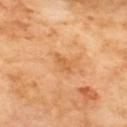follow-up: total-body-photography surveillance lesion; no biopsy | acquisition: ~15 mm tile from a whole-body skin photo | illumination: cross-polarized illumination | anatomic site: the back.